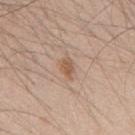Impression: The lesion was tiled from a total-body skin photograph and was not biopsied. Clinical summary: From the mid back. A region of skin cropped from a whole-body photographic capture, roughly 15 mm wide. A male patient, roughly 50 years of age.